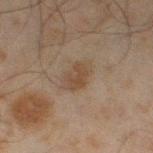Captured during whole-body skin photography for melanoma surveillance; the lesion was not biopsied. The tile uses cross-polarized illumination. A lesion tile, about 15 mm wide, cut from a 3D total-body photograph. A male patient aged around 45. From the left thigh.